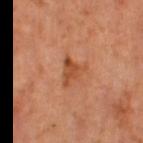Part of a total-body skin-imaging series; this lesion was reviewed on a skin check and was not flagged for biopsy. A lesion tile, about 15 mm wide, cut from a 3D total-body photograph. A female subject, about 65 years old. The lesion's longest dimension is about 3.5 mm. The lesion is located on the left thigh.